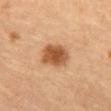The lesion was photographed on a routine skin check and not biopsied; there is no pathology result. A lesion tile, about 15 mm wide, cut from a 3D total-body photograph. The lesion is located on the abdomen. Approximately 4 mm at its widest. The tile uses cross-polarized illumination. A female subject aged 58 to 62.